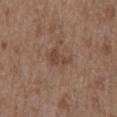workup — total-body-photography surveillance lesion; no biopsy | TBP lesion metrics — an area of roughly 3.5 mm², an outline eccentricity of about 0.85 (0 = round, 1 = elongated), and two-axis asymmetry of about 0.6; an average lesion color of about L≈43 a*≈18 b*≈26 (CIELAB), about 8 CIELAB-L* units darker than the surrounding skin, and a normalized border contrast of about 6.5; a border-irregularity rating of about 7/10, internal color variation of about 1.5 on a 0–10 scale, and radial color variation of about 0.5 | imaging modality — ~15 mm crop, total-body skin-cancer survey | patient — male, aged around 50 | tile lighting — white-light illumination | lesion size — ≈3 mm | anatomic site — the mid back.